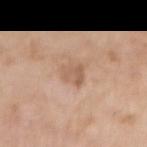Assessment:
No biopsy was performed on this lesion — it was imaged during a full skin examination and was not determined to be concerning.
Context:
The patient is a male in their 60s. A region of skin cropped from a whole-body photographic capture, roughly 15 mm wide. The lesion is located on the right upper arm. This is a white-light tile. An algorithmic analysis of the crop reported a classifier nevus-likeness of about 5/100 and lesion-presence confidence of about 100/100.